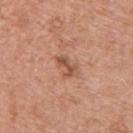Q: Is there a histopathology result?
A: no biopsy performed (imaged during a skin exam)
Q: What kind of image is this?
A: ~15 mm tile from a whole-body skin photo
Q: What is the anatomic site?
A: the back
Q: Patient demographics?
A: male, about 80 years old